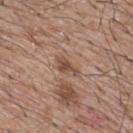| field | value |
|---|---|
| notes | imaged on a skin check; not biopsied |
| illumination | white-light |
| image source | 15 mm crop, total-body photography |
| automated lesion analysis | a lesion color around L≈48 a*≈19 b*≈27 in CIELAB, about 10 CIELAB-L* units darker than the surrounding skin, and a lesion-to-skin contrast of about 7.5 (normalized; higher = more distinct) |
| body site | the upper back |
| patient | male, aged around 65 |
| lesion diameter | about 3 mm |